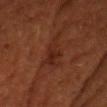{"automated_metrics": {"cielab_L": 24, "cielab_a": 22, "cielab_b": 26, "vs_skin_darker_L": 6.0, "vs_skin_contrast_norm": 6.5, "border_irregularity_0_10": 6.0}, "site": "head or neck", "image": {"source": "total-body photography crop", "field_of_view_mm": 15}, "patient": {"sex": "male", "age_approx": 55}, "lighting": "cross-polarized"}